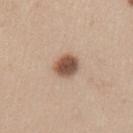Findings:
- patient — male, in their mid- to late 30s
- image — 15 mm crop, total-body photography
- illumination — white-light illumination
- site — the left upper arm